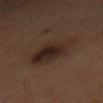No biopsy was performed on this lesion — it was imaged during a full skin examination and was not determined to be concerning.
A female subject, approximately 60 years of age.
A lesion tile, about 15 mm wide, cut from a 3D total-body photograph.
An algorithmic analysis of the crop reported an area of roughly 16 mm², an eccentricity of roughly 0.9, and a shape-asymmetry score of about 0.3 (0 = symmetric). The software also gave a border-irregularity index near 4.5/10, a within-lesion color-variation index near 5/10, and peripheral color asymmetry of about 1.5. It also reported a nevus-likeness score of about 90/100 and lesion-presence confidence of about 100/100.
Longest diameter approximately 7 mm.
Imaged with cross-polarized lighting.
The lesion is located on the mid back.